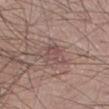Q: Was a biopsy performed?
A: total-body-photography surveillance lesion; no biopsy
Q: What did automated image analysis measure?
A: an eccentricity of roughly 0.7 and a symmetry-axis asymmetry near 0.35; a lesion–skin lightness drop of about 7 and a lesion-to-skin contrast of about 6 (normalized; higher = more distinct); border irregularity of about 3.5 on a 0–10 scale and peripheral color asymmetry of about 1; a classifier nevus-likeness of about 0/100
Q: What kind of image is this?
A: total-body-photography crop, ~15 mm field of view
Q: What are the patient's age and sex?
A: male, approximately 55 years of age